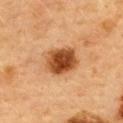Notes:
• biopsy status · total-body-photography surveillance lesion; no biopsy
• site · the upper back
• TBP lesion metrics · an eccentricity of roughly 0.6 and a shape-asymmetry score of about 0.1 (0 = symmetric); a lesion-to-skin contrast of about 12 (normalized; higher = more distinct); a nevus-likeness score of about 100/100 and a lesion-detection confidence of about 100/100
• lesion diameter · ~4.5 mm (longest diameter)
• acquisition · 15 mm crop, total-body photography
• patient · female, aged 58 to 62